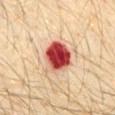Imaged during a routine full-body skin examination; the lesion was not biopsied and no histopathology is available. A close-up tile cropped from a whole-body skin photograph, about 15 mm across. A male patient approximately 30 years of age. The recorded lesion diameter is about 4.5 mm. The lesion is located on the chest. The tile uses cross-polarized illumination.